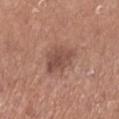Captured during whole-body skin photography for melanoma surveillance; the lesion was not biopsied. A 15 mm close-up tile from a total-body photography series done for melanoma screening. The lesion is located on the leg. The subject is a female approximately 65 years of age.